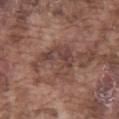Assessment:
Recorded during total-body skin imaging; not selected for excision or biopsy.
Image and clinical context:
On the left thigh. Automated image analysis of the tile measured a mean CIELAB color near L≈42 a*≈19 b*≈22, roughly 8 lightness units darker than nearby skin, and a normalized lesion–skin contrast near 7. A male subject aged approximately 75. Cropped from a whole-body photographic skin survey; the tile spans about 15 mm.